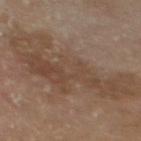Imaged during a routine full-body skin examination; the lesion was not biopsied and no histopathology is available. From the mid back. Cropped from a whole-body photographic skin survey; the tile spans about 15 mm. Longest diameter approximately 13.5 mm. A male subject, aged around 70.